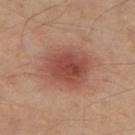Clinical impression:
Part of a total-body skin-imaging series; this lesion was reviewed on a skin check and was not flagged for biopsy.
Acquisition and patient details:
Cropped from a whole-body photographic skin survey; the tile spans about 15 mm. On the left thigh. Imaged with cross-polarized lighting. A male patient, approximately 55 years of age.